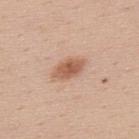Acquisition and patient details:
Automated tile analysis of the lesion measured a footprint of about 6 mm², an outline eccentricity of about 0.85 (0 = round, 1 = elongated), and two-axis asymmetry of about 0.15. And it measured internal color variation of about 3 on a 0–10 scale and a peripheral color-asymmetry measure near 1. Located on the upper back. A 15 mm close-up extracted from a 3D total-body photography capture. The recorded lesion diameter is about 4 mm. The subject is a male aged 38–42.